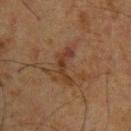The lesion was tiled from a total-body skin photograph and was not biopsied.
The patient is a male aged approximately 60.
From the back.
A 15 mm crop from a total-body photograph taken for skin-cancer surveillance.
The tile uses cross-polarized illumination.
Measured at roughly 4.5 mm in maximum diameter.